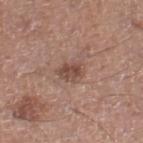biopsy status: catalogued during a skin exam; not biopsied | image source: total-body-photography crop, ~15 mm field of view | anatomic site: the right lower leg | patient: male, about 55 years old.